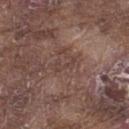The lesion-visualizer software estimated a lesion area of about 3 mm², an outline eccentricity of about 0.9 (0 = round, 1 = elongated), and two-axis asymmetry of about 0.6.
The tile uses white-light illumination.
On the left thigh.
Longest diameter approximately 3 mm.
Cropped from a whole-body photographic skin survey; the tile spans about 15 mm.
A male patient, in their mid-70s.